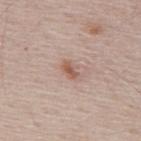The patient is a male roughly 50 years of age. A close-up tile cropped from a whole-body skin photograph, about 15 mm across. Located on the upper back.